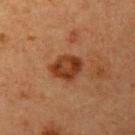biopsy status = no biopsy performed (imaged during a skin exam); body site = the left upper arm; acquisition = total-body-photography crop, ~15 mm field of view; lighting = cross-polarized; diameter = ≈4 mm; patient = female, aged 38 to 42.